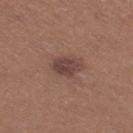This lesion was catalogued during total-body skin photography and was not selected for biopsy. Imaged with white-light lighting. The patient is a female in their 40s. The recorded lesion diameter is about 3.5 mm. Automated tile analysis of the lesion measured a footprint of about 6 mm², a shape eccentricity near 0.7, and a symmetry-axis asymmetry near 0.25. It also reported an average lesion color of about L≈42 a*≈19 b*≈22 (CIELAB) and a lesion–skin lightness drop of about 10. It also reported a border-irregularity index near 2.5/10, a within-lesion color-variation index near 3/10, and peripheral color asymmetry of about 1. The software also gave a lesion-detection confidence of about 100/100. From the right thigh. A 15 mm crop from a total-body photograph taken for skin-cancer surveillance.